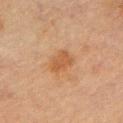biopsy status — catalogued during a skin exam; not biopsied | location — the right upper arm | imaging modality — ~15 mm crop, total-body skin-cancer survey | patient — male, in their mid- to late 60s.